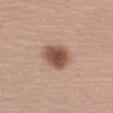Recorded during total-body skin imaging; not selected for excision or biopsy. A male patient approximately 65 years of age. The tile uses white-light illumination. From the right upper arm. Approximately 4.5 mm at its widest. A 15 mm crop from a total-body photograph taken for skin-cancer surveillance. Automated image analysis of the tile measured a normalized border contrast of about 10. The software also gave internal color variation of about 4 on a 0–10 scale and peripheral color asymmetry of about 1.